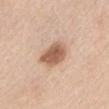Assessment:
The lesion was photographed on a routine skin check and not biopsied; there is no pathology result.
Background:
Cropped from a whole-body photographic skin survey; the tile spans about 15 mm. From the chest. The patient is a female aged 63–67.